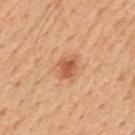Case summary:
• patient: male, aged approximately 50
• lesion size: ~2.5 mm (longest diameter)
• lighting: white-light illumination
• anatomic site: the mid back
• automated lesion analysis: a lesion-to-skin contrast of about 7.5 (normalized; higher = more distinct); a nevus-likeness score of about 90/100 and lesion-presence confidence of about 100/100
• acquisition: total-body-photography crop, ~15 mm field of view